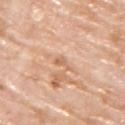workup — no biopsy performed (imaged during a skin exam)
body site — the upper back
size — ≈3 mm
illumination — white-light
image — ~15 mm crop, total-body skin-cancer survey
patient — male, in their mid-70s